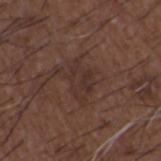{"biopsy_status": "not biopsied; imaged during a skin examination", "image": {"source": "total-body photography crop", "field_of_view_mm": 15}, "lesion_size": {"long_diameter_mm_approx": 4.0}, "lighting": "white-light", "patient": {"sex": "male", "age_approx": 50}, "site": "back", "automated_metrics": {"area_mm2_approx": 7.5, "eccentricity": 0.7, "shape_asymmetry": 0.3, "cielab_L": 31, "cielab_a": 17, "cielab_b": 21, "vs_skin_darker_L": 5.0, "vs_skin_contrast_norm": 6.0, "border_irregularity_0_10": 4.0, "color_variation_0_10": 2.5}}